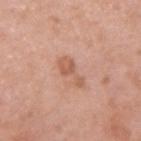Q: What kind of image is this?
A: ~15 mm crop, total-body skin-cancer survey
Q: Who is the patient?
A: female, aged approximately 40
Q: What is the anatomic site?
A: the arm